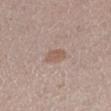Case summary:
* workup — no biopsy performed (imaged during a skin exam)
* automated lesion analysis — a footprint of about 4 mm², an outline eccentricity of about 0.8 (0 = round, 1 = elongated), and two-axis asymmetry of about 0.15; a mean CIELAB color near L≈56 a*≈17 b*≈25, roughly 8 lightness units darker than nearby skin, and a lesion-to-skin contrast of about 6 (normalized; higher = more distinct); border irregularity of about 1.5 on a 0–10 scale and a peripheral color-asymmetry measure near 0.5; a nevus-likeness score of about 60/100
* image source — ~15 mm crop, total-body skin-cancer survey
* size — ≈2.5 mm
* subject — female, aged 18 to 22
* lighting — white-light
* site — the left lower leg